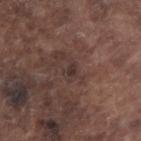{"biopsy_status": "not biopsied; imaged during a skin examination", "patient": {"sex": "male", "age_approx": 75}, "lesion_size": {"long_diameter_mm_approx": 2.5}, "site": "arm", "image": {"source": "total-body photography crop", "field_of_view_mm": 15}, "automated_metrics": {"eccentricity": 0.8, "cielab_L": 33, "cielab_a": 16, "cielab_b": 18, "vs_skin_contrast_norm": 6.5, "border_irregularity_0_10": 5.0, "color_variation_0_10": 0.0}, "lighting": "white-light"}